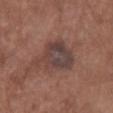Findings:
- follow-up · total-body-photography surveillance lesion; no biopsy
- image · total-body-photography crop, ~15 mm field of view
- size · ≈5.5 mm
- patient · female, in their mid-70s
- body site · the upper back